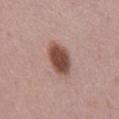| field | value |
|---|---|
| workup | imaged on a skin check; not biopsied |
| image | 15 mm crop, total-body photography |
| lesion size | ~4.5 mm (longest diameter) |
| site | the lower back |
| illumination | white-light illumination |
| patient | male, approximately 50 years of age |